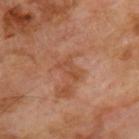<case>
  <biopsy_status>not biopsied; imaged during a skin examination</biopsy_status>
  <site>upper back</site>
  <lesion_size>
    <long_diameter_mm_approx>3.0</long_diameter_mm_approx>
  </lesion_size>
  <image>
    <source>total-body photography crop</source>
    <field_of_view_mm>15</field_of_view_mm>
  </image>
  <lighting>cross-polarized</lighting>
  <patient>
    <sex>male</sex>
    <age_approx>70</age_approx>
  </patient>
</case>